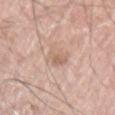Impression: No biopsy was performed on this lesion — it was imaged during a full skin examination and was not determined to be concerning. Context: A region of skin cropped from a whole-body photographic capture, roughly 15 mm wide. This is a white-light tile. A male subject, approximately 65 years of age. About 4 mm across. Located on the left upper arm.